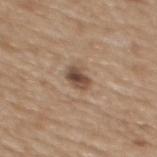Assessment: Captured during whole-body skin photography for melanoma surveillance; the lesion was not biopsied. Acquisition and patient details: This image is a 15 mm lesion crop taken from a total-body photograph. Captured under white-light illumination. The subject is a female aged approximately 70. Longest diameter approximately 2.5 mm. The lesion is located on the upper back. Automated tile analysis of the lesion measured peripheral color asymmetry of about 2. The software also gave an automated nevus-likeness rating near 75 out of 100 and lesion-presence confidence of about 100/100.